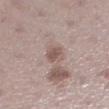The lesion was photographed on a routine skin check and not biopsied; there is no pathology result. Longest diameter approximately 2.5 mm. A female subject, in their mid-50s. Automated tile analysis of the lesion measured a lesion area of about 4 mm², an eccentricity of roughly 0.6, and two-axis asymmetry of about 0.25. It also reported a border-irregularity index near 2.5/10 and a peripheral color-asymmetry measure near 0.5. The software also gave a classifier nevus-likeness of about 30/100 and lesion-presence confidence of about 100/100. A roughly 15 mm field-of-view crop from a total-body skin photograph. The lesion is located on the left lower leg. Imaged with white-light lighting.